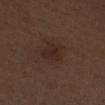Q: Was this lesion biopsied?
A: total-body-photography surveillance lesion; no biopsy
Q: Lesion location?
A: the right thigh
Q: What is the imaging modality?
A: total-body-photography crop, ~15 mm field of view
Q: What are the patient's age and sex?
A: male, roughly 70 years of age
Q: Automated lesion metrics?
A: a classifier nevus-likeness of about 35/100